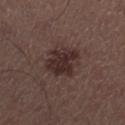notes: no biopsy performed (imaged during a skin exam)
body site: the right lower leg
patient: male, aged 53–57
imaging modality: ~15 mm crop, total-body skin-cancer survey
lighting: white-light